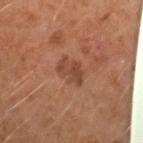Q: Is there a histopathology result?
A: total-body-photography surveillance lesion; no biopsy
Q: What lighting was used for the tile?
A: cross-polarized illumination
Q: Automated lesion metrics?
A: a footprint of about 7 mm² and a symmetry-axis asymmetry near 0.3; an average lesion color of about L≈41 a*≈21 b*≈29 (CIELAB), about 8 CIELAB-L* units darker than the surrounding skin, and a lesion-to-skin contrast of about 6.5 (normalized; higher = more distinct); border irregularity of about 3.5 on a 0–10 scale, a color-variation rating of about 3/10, and a peripheral color-asymmetry measure near 1; a classifier nevus-likeness of about 0/100
Q: What is the lesion's diameter?
A: ≈4 mm
Q: Who is the patient?
A: male, aged around 60
Q: How was this image acquired?
A: 15 mm crop, total-body photography
Q: Lesion location?
A: the left forearm